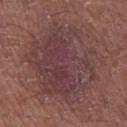<lesion>
  <biopsy_status>not biopsied; imaged during a skin examination</biopsy_status>
  <patient>
    <sex>male</sex>
    <age_approx>55</age_approx>
  </patient>
  <lesion_size>
    <long_diameter_mm_approx>10.0</long_diameter_mm_approx>
  </lesion_size>
  <lighting>white-light</lighting>
  <image>
    <source>total-body photography crop</source>
    <field_of_view_mm>15</field_of_view_mm>
  </image>
  <automated_metrics>
    <cielab_L>39</cielab_L>
    <cielab_a>20</cielab_a>
    <cielab_b>17</cielab_b>
    <vs_skin_darker_L>7.0</vs_skin_darker_L>
    <vs_skin_contrast_norm>7.5</vs_skin_contrast_norm>
    <color_variation_0_10>5.0</color_variation_0_10>
    <peripheral_color_asymmetry>1.5</peripheral_color_asymmetry>
    <nevus_likeness_0_100>0</nevus_likeness_0_100>
    <lesion_detection_confidence_0_100>95</lesion_detection_confidence_0_100>
  </automated_metrics>
  <site>left thigh</site>
</lesion>